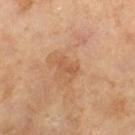Clinical impression: Recorded during total-body skin imaging; not selected for excision or biopsy. Context: This image is a 15 mm lesion crop taken from a total-body photograph. About 2.5 mm across. Captured under cross-polarized illumination. A male subject, aged around 65.